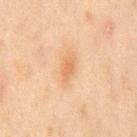Assessment:
Captured during whole-body skin photography for melanoma surveillance; the lesion was not biopsied.
Context:
On the chest. The total-body-photography lesion software estimated a lesion color around L≈55 a*≈19 b*≈34 in CIELAB, a lesion–skin lightness drop of about 7, and a normalized border contrast of about 5.5. It also reported internal color variation of about 1 on a 0–10 scale and peripheral color asymmetry of about 0.5. It also reported lesion-presence confidence of about 100/100. A male patient about 45 years old. A 15 mm close-up extracted from a 3D total-body photography capture. The lesion's longest dimension is about 3 mm. This is a cross-polarized tile.